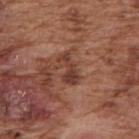Notes:
* acquisition — 15 mm crop, total-body photography
* subject — male, roughly 75 years of age
* automated lesion analysis — an average lesion color of about L≈39 a*≈23 b*≈27 (CIELAB), about 10 CIELAB-L* units darker than the surrounding skin, and a lesion-to-skin contrast of about 8.5 (normalized; higher = more distinct)
* lighting — white-light
* location — the right upper arm